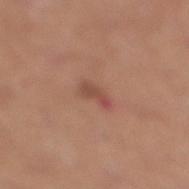Q: Is there a histopathology result?
A: imaged on a skin check; not biopsied
Q: Where on the body is the lesion?
A: the leg
Q: What is the imaging modality?
A: ~15 mm tile from a whole-body skin photo
Q: Who is the patient?
A: male, in their mid-70s